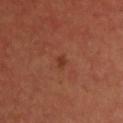Impression: Captured during whole-body skin photography for melanoma surveillance; the lesion was not biopsied. Image and clinical context: The patient is a male roughly 65 years of age. Captured under cross-polarized illumination. A lesion tile, about 15 mm wide, cut from a 3D total-body photograph. Approximately 1.5 mm at its widest. From the chest.